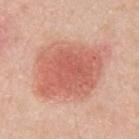<tbp_lesion>
<biopsy_status>not biopsied; imaged during a skin examination</biopsy_status>
<lighting>white-light</lighting>
<automated_metrics>
  <area_mm2_approx>41.0</area_mm2_approx>
  <eccentricity>0.65</eccentricity>
  <shape_asymmetry>0.2</shape_asymmetry>
  <cielab_L>61</cielab_L>
  <cielab_a>28</cielab_a>
  <cielab_b>30</cielab_b>
  <vs_skin_darker_L>12.0</vs_skin_darker_L>
  <vs_skin_contrast_norm>7.5</vs_skin_contrast_norm>
  <nevus_likeness_0_100>100</nevus_likeness_0_100>
  <lesion_detection_confidence_0_100>100</lesion_detection_confidence_0_100>
</automated_metrics>
<lesion_size>
  <long_diameter_mm_approx>8.0</long_diameter_mm_approx>
</lesion_size>
<patient>
  <sex>male</sex>
  <age_approx>45</age_approx>
</patient>
<image>
  <source>total-body photography crop</source>
  <field_of_view_mm>15</field_of_view_mm>
</image>
<site>chest</site>
</tbp_lesion>